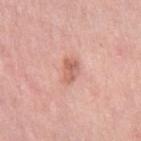No biopsy was performed on this lesion — it was imaged during a full skin examination and was not determined to be concerning. The lesion's longest dimension is about 3 mm. A 15 mm crop from a total-body photograph taken for skin-cancer surveillance. The patient is a female aged 38–42. Located on the right thigh. Captured under white-light illumination.